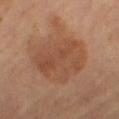From the right thigh.
A female subject, aged 68 to 72.
The tile uses cross-polarized illumination.
A lesion tile, about 15 mm wide, cut from a 3D total-body photograph.
Automated image analysis of the tile measured an area of roughly 30 mm², an eccentricity of roughly 0.6, and a shape-asymmetry score of about 0.2 (0 = symmetric). The software also gave lesion-presence confidence of about 100/100.